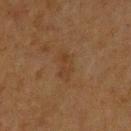notes: catalogued during a skin exam; not biopsied
lesion size: ≈4 mm
patient: male, aged around 75
lighting: cross-polarized
imaging modality: 15 mm crop, total-body photography
site: the upper back